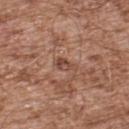| key | value |
|---|---|
| follow-up | imaged on a skin check; not biopsied |
| patient | male, in their mid-50s |
| automated lesion analysis | a footprint of about 3 mm² and an eccentricity of roughly 0.85; internal color variation of about 3 on a 0–10 scale and radial color variation of about 1; a classifier nevus-likeness of about 0/100 and a detector confidence of about 100 out of 100 that the crop contains a lesion |
| body site | the back |
| lighting | white-light illumination |
| image source | total-body-photography crop, ~15 mm field of view |